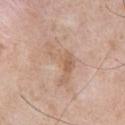Q: Was this lesion biopsied?
A: imaged on a skin check; not biopsied
Q: Who is the patient?
A: male, about 50 years old
Q: What is the anatomic site?
A: the chest
Q: Illumination type?
A: white-light illumination
Q: Automated lesion metrics?
A: a lesion area of about 8.5 mm² and a symmetry-axis asymmetry near 0.8; a lesion color around L≈61 a*≈18 b*≈30 in CIELAB, roughly 7 lightness units darker than nearby skin, and a normalized lesion–skin contrast near 5.5; a detector confidence of about 100 out of 100 that the crop contains a lesion
Q: How was this image acquired?
A: 15 mm crop, total-body photography
Q: Lesion size?
A: ~5.5 mm (longest diameter)